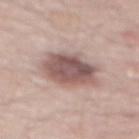workup: no biopsy performed (imaged during a skin exam) | imaging modality: 15 mm crop, total-body photography | body site: the back | subject: male, aged approximately 70.